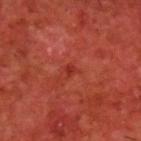Notes:
* imaging modality — ~15 mm tile from a whole-body skin photo
* patient — male, aged 58 to 62
* lesion diameter — about 1 mm
* automated metrics — an area of roughly 1 mm², an eccentricity of roughly 0.6, and a shape-asymmetry score of about 0.3 (0 = symmetric); about 7 CIELAB-L* units darker than the surrounding skin and a lesion-to-skin contrast of about 6 (normalized; higher = more distinct)
* body site — the upper back